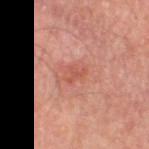Recorded during total-body skin imaging; not selected for excision or biopsy.
The lesion's longest dimension is about 2.5 mm.
The lesion is on the leg.
Imaged with cross-polarized lighting.
A region of skin cropped from a whole-body photographic capture, roughly 15 mm wide.
The subject is a male in their 70s.